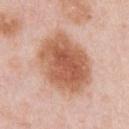Recorded during total-body skin imaging; not selected for excision or biopsy. The lesion is located on the chest. Approximately 7.5 mm at its widest. Captured under white-light illumination. Automated tile analysis of the lesion measured an outline eccentricity of about 0.6 (0 = round, 1 = elongated) and two-axis asymmetry of about 0.15. A 15 mm close-up tile from a total-body photography series done for melanoma screening. A male patient in their 60s.